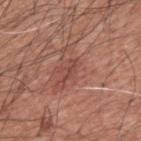workup: no biopsy performed (imaged during a skin exam)
anatomic site: the back
image-analysis metrics: an area of roughly 2.5 mm² and an outline eccentricity of about 0.95 (0 = round, 1 = elongated); a mean CIELAB color near L≈45 a*≈25 b*≈25; a color-variation rating of about 0/10 and a peripheral color-asymmetry measure near 0; lesion-presence confidence of about 70/100
tile lighting: white-light
subject: male, about 60 years old
imaging modality: 15 mm crop, total-body photography